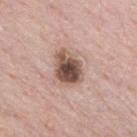| feature | finding |
|---|---|
| biopsy status | total-body-photography surveillance lesion; no biopsy |
| subject | male, about 60 years old |
| anatomic site | the chest |
| acquisition | ~15 mm crop, total-body skin-cancer survey |
| lesion size | about 4 mm |